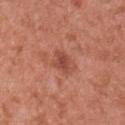Clinical impression: The lesion was photographed on a routine skin check and not biopsied; there is no pathology result. Background: This is a white-light tile. A female subject aged 33–37. This image is a 15 mm lesion crop taken from a total-body photograph. An algorithmic analysis of the crop reported a footprint of about 5 mm² and two-axis asymmetry of about 0.25. The analysis additionally found a border-irregularity rating of about 3/10 and radial color variation of about 0.5. About 3 mm across. From the back.